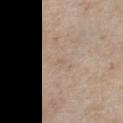Q: Was this lesion biopsied?
A: imaged on a skin check; not biopsied
Q: What are the patient's age and sex?
A: male, in their mid-50s
Q: Where on the body is the lesion?
A: the front of the torso
Q: How was this image acquired?
A: total-body-photography crop, ~15 mm field of view
Q: How was the tile lit?
A: white-light
Q: How large is the lesion?
A: ≈1 mm